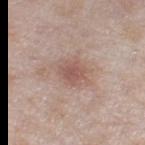Q: Was this lesion biopsied?
A: imaged on a skin check; not biopsied
Q: What lighting was used for the tile?
A: white-light
Q: Lesion size?
A: ~3 mm (longest diameter)
Q: How was this image acquired?
A: total-body-photography crop, ~15 mm field of view
Q: Automated lesion metrics?
A: an average lesion color of about L≈55 a*≈19 b*≈24 (CIELAB), roughly 9 lightness units darker than nearby skin, and a normalized border contrast of about 6; a border-irregularity index near 2.5/10, internal color variation of about 2 on a 0–10 scale, and peripheral color asymmetry of about 0.5
Q: What are the patient's age and sex?
A: female, aged approximately 60
Q: Where on the body is the lesion?
A: the right thigh